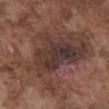Imaged during a routine full-body skin examination; the lesion was not biopsied and no histopathology is available.
Automated image analysis of the tile measured an area of roughly 55 mm², an outline eccentricity of about 0.8 (0 = round, 1 = elongated), and a symmetry-axis asymmetry near 0.4. The software also gave border irregularity of about 8 on a 0–10 scale and radial color variation of about 2.
A 15 mm crop from a total-body photograph taken for skin-cancer surveillance.
The lesion is on the abdomen.
The tile uses white-light illumination.
Approximately 13 mm at its widest.
The subject is a male in their mid- to late 70s.